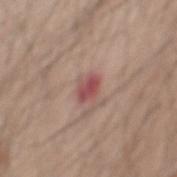  biopsy_status: not biopsied; imaged during a skin examination
  image:
    source: total-body photography crop
    field_of_view_mm: 15
  site: mid back
  automated_metrics:
    area_mm2_approx: 4.0
    eccentricity: 0.75
    shape_asymmetry: 0.2
    border_irregularity_0_10: 2.0
    peripheral_color_asymmetry: 1.5
    nevus_likeness_0_100: 0
    lesion_detection_confidence_0_100: 100
  lesion_size:
    long_diameter_mm_approx: 3.0
  lighting: white-light
  patient:
    sex: male
    age_approx: 60